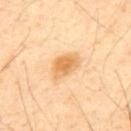Background:
A male patient, in their 50s. The lesion is on the mid back. Imaged with cross-polarized lighting. Automated image analysis of the tile measured a lesion area of about 7.5 mm², an eccentricity of roughly 0.75, and two-axis asymmetry of about 0.25. The analysis additionally found a lesion color around L≈71 a*≈21 b*≈46 in CIELAB, a lesion–skin lightness drop of about 11, and a lesion-to-skin contrast of about 8 (normalized; higher = more distinct). The software also gave internal color variation of about 4 on a 0–10 scale and peripheral color asymmetry of about 1. And it measured a lesion-detection confidence of about 100/100. Approximately 4 mm at its widest. A 15 mm crop from a total-body photograph taken for skin-cancer surveillance.